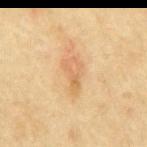biopsy status = no biopsy performed (imaged during a skin exam).